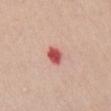notes: catalogued during a skin exam; not biopsied
patient: female, aged approximately 70
size: about 2.5 mm
TBP lesion metrics: an outline eccentricity of about 0.6 (0 = round, 1 = elongated) and a shape-asymmetry score of about 0.25 (0 = symmetric); a normalized border contrast of about 10; a color-variation rating of about 3.5/10 and a peripheral color-asymmetry measure near 1
location: the chest
acquisition: ~15 mm tile from a whole-body skin photo
tile lighting: white-light